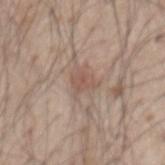Imaged during a routine full-body skin examination; the lesion was not biopsied and no histopathology is available. A 15 mm close-up extracted from a 3D total-body photography capture. The lesion is located on the mid back. A male patient about 50 years old.